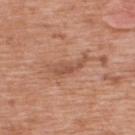{"biopsy_status": "not biopsied; imaged during a skin examination", "site": "upper back", "lesion_size": {"long_diameter_mm_approx": 5.0}, "lighting": "white-light", "image": {"source": "total-body photography crop", "field_of_view_mm": 15}, "patient": {"sex": "male", "age_approx": 70}}